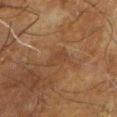Part of a total-body skin-imaging series; this lesion was reviewed on a skin check and was not flagged for biopsy. The tile uses cross-polarized illumination. A 15 mm close-up extracted from a 3D total-body photography capture. The lesion is located on the leg. The lesion's longest dimension is about 2.5 mm. The subject is a male in their mid-60s. The lesion-visualizer software estimated an automated nevus-likeness rating near 0 out of 100 and lesion-presence confidence of about 90/100.